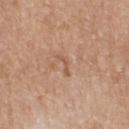Part of a total-body skin-imaging series; this lesion was reviewed on a skin check and was not flagged for biopsy.
A male subject, aged 68–72.
From the upper back.
Cropped from a whole-body photographic skin survey; the tile spans about 15 mm.